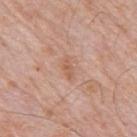Impression: No biopsy was performed on this lesion — it was imaged during a full skin examination and was not determined to be concerning. Context: Located on the mid back. A male patient, approximately 80 years of age. About 3 mm across. Captured under white-light illumination. A 15 mm crop from a total-body photograph taken for skin-cancer surveillance. An algorithmic analysis of the crop reported a nevus-likeness score of about 0/100 and a lesion-detection confidence of about 100/100.